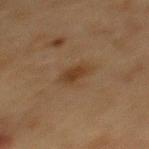{
  "biopsy_status": "not biopsied; imaged during a skin examination",
  "site": "back",
  "patient": {
    "sex": "female",
    "age_approx": 40
  },
  "lighting": "cross-polarized",
  "automated_metrics": {
    "area_mm2_approx": 5.0,
    "eccentricity": 0.8,
    "shape_asymmetry": 0.25,
    "cielab_L": 33,
    "cielab_a": 15,
    "cielab_b": 28,
    "vs_skin_contrast_norm": 7.0,
    "border_irregularity_0_10": 2.5,
    "color_variation_0_10": 2.0,
    "peripheral_color_asymmetry": 0.5
  },
  "image": {
    "source": "total-body photography crop",
    "field_of_view_mm": 15
  }
}